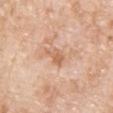Part of a total-body skin-imaging series; this lesion was reviewed on a skin check and was not flagged for biopsy.
Cropped from a whole-body photographic skin survey; the tile spans about 15 mm.
A male subject roughly 80 years of age.
From the chest.